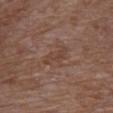This lesion was catalogued during total-body skin photography and was not selected for biopsy.
A female patient roughly 80 years of age.
A 15 mm close-up tile from a total-body photography series done for melanoma screening.
From the chest.
About 4 mm across.
Imaged with white-light lighting.